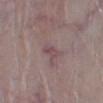| key | value |
|---|---|
| follow-up | catalogued during a skin exam; not biopsied |
| anatomic site | the left lower leg |
| image | ~15 mm crop, total-body skin-cancer survey |
| lesion size | ~3 mm (longest diameter) |
| patient | male, in their mid-60s |